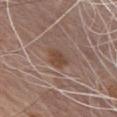The lesion was tiled from a total-body skin photograph and was not biopsied. On the chest. A region of skin cropped from a whole-body photographic capture, roughly 15 mm wide. This is a white-light tile. The recorded lesion diameter is about 3 mm. The total-body-photography lesion software estimated a footprint of about 5 mm², an outline eccentricity of about 0.8 (0 = round, 1 = elongated), and two-axis asymmetry of about 0.3. It also reported a lesion color around L≈45 a*≈18 b*≈26 in CIELAB and a normalized border contrast of about 7.5. The software also gave border irregularity of about 3 on a 0–10 scale, a color-variation rating of about 2/10, and peripheral color asymmetry of about 0.5. And it measured an automated nevus-likeness rating near 85 out of 100 and lesion-presence confidence of about 100/100. A male subject aged approximately 70.